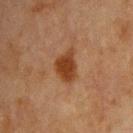– follow-up: total-body-photography surveillance lesion; no biopsy
– TBP lesion metrics: a lesion area of about 8 mm², an eccentricity of roughly 0.65, and two-axis asymmetry of about 0.3
– subject: male, about 65 years old
– body site: the chest
– illumination: cross-polarized
– imaging modality: 15 mm crop, total-body photography
– size: ~4 mm (longest diameter)